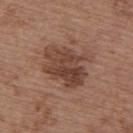Clinical impression: The lesion was photographed on a routine skin check and not biopsied; there is no pathology result. Acquisition and patient details: On the upper back. A region of skin cropped from a whole-body photographic capture, roughly 15 mm wide. A female patient, in their mid-60s.